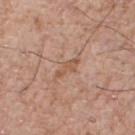Assessment:
Part of a total-body skin-imaging series; this lesion was reviewed on a skin check and was not flagged for biopsy.
Image and clinical context:
A male patient aged approximately 75. Imaged with white-light lighting. From the chest. Longest diameter approximately 3.5 mm. Cropped from a whole-body photographic skin survey; the tile spans about 15 mm.